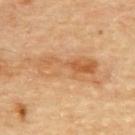Q: Was a biopsy performed?
A: no biopsy performed (imaged during a skin exam)
Q: How was the tile lit?
A: cross-polarized
Q: What kind of image is this?
A: ~15 mm tile from a whole-body skin photo
Q: Lesion location?
A: the upper back
Q: Patient demographics?
A: male, aged approximately 85 or older